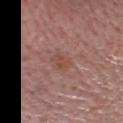Acquisition and patient details:
Longest diameter approximately 3 mm. The lesion is on the head or neck. Cropped from a total-body skin-imaging series; the visible field is about 15 mm. The subject is a male approximately 75 years of age.